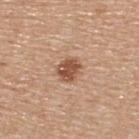Clinical impression: The lesion was tiled from a total-body skin photograph and was not biopsied. Background: The recorded lesion diameter is about 3 mm. Imaged with white-light lighting. A male patient, aged 53–57. Automated tile analysis of the lesion measured a footprint of about 5.5 mm² and an eccentricity of roughly 0.5. The analysis additionally found roughly 13 lightness units darker than nearby skin. Cropped from a whole-body photographic skin survey; the tile spans about 15 mm. Located on the upper back.